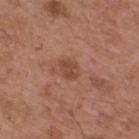Imaged with white-light lighting. From the upper back. A male subject aged 53–57. A 15 mm close-up tile from a total-body photography series done for melanoma screening. Measured at roughly 2.5 mm in maximum diameter.